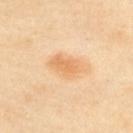Assessment: Recorded during total-body skin imaging; not selected for excision or biopsy. Background: The subject is a female aged 38 to 42. Longest diameter approximately 4 mm. A 15 mm close-up tile from a total-body photography series done for melanoma screening. On the upper back.